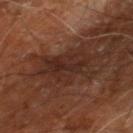Q: Is there a histopathology result?
A: catalogued during a skin exam; not biopsied
Q: What is the imaging modality?
A: 15 mm crop, total-body photography
Q: Where on the body is the lesion?
A: the right leg
Q: Who is the patient?
A: male, aged around 60